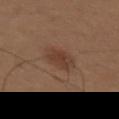follow-up = catalogued during a skin exam; not biopsied | subject = male, aged around 75 | lighting = white-light illumination | location = the upper back | image = total-body-photography crop, ~15 mm field of view | diameter = ≈4.5 mm.